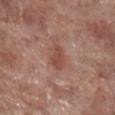follow-up: imaged on a skin check; not biopsied
subject: male, about 70 years old
tile lighting: white-light illumination
imaging modality: ~15 mm crop, total-body skin-cancer survey
site: the right lower leg